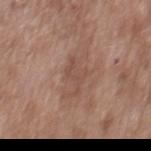follow-up = no biopsy performed (imaged during a skin exam)
patient = male, about 50 years old
acquisition = 15 mm crop, total-body photography
lighting = white-light illumination
location = the mid back